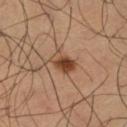Assessment:
Recorded during total-body skin imaging; not selected for excision or biopsy.
Clinical summary:
Located on the left thigh. Automated tile analysis of the lesion measured a footprint of about 6 mm² and an eccentricity of roughly 0.75. The software also gave a border-irregularity rating of about 3/10. The software also gave a nevus-likeness score of about 95/100. Imaged with cross-polarized lighting. The subject is a male aged 63 to 67. The lesion's longest dimension is about 3.5 mm. Cropped from a total-body skin-imaging series; the visible field is about 15 mm.